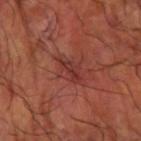Q: Was a biopsy performed?
A: no biopsy performed (imaged during a skin exam)